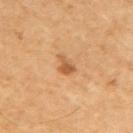{
  "biopsy_status": "not biopsied; imaged during a skin examination",
  "lesion_size": {
    "long_diameter_mm_approx": 2.5
  },
  "lighting": "cross-polarized",
  "automated_metrics": {
    "eccentricity": 0.8,
    "shape_asymmetry": 0.45,
    "cielab_L": 55,
    "cielab_a": 23,
    "cielab_b": 40,
    "vs_skin_darker_L": 11.0,
    "vs_skin_contrast_norm": 7.5,
    "border_irregularity_0_10": 4.0,
    "nevus_likeness_0_100": 60,
    "lesion_detection_confidence_0_100": 100
  },
  "image": {
    "source": "total-body photography crop",
    "field_of_view_mm": 15
  },
  "patient": {
    "sex": "male",
    "age_approx": 60
  },
  "site": "upper back"
}